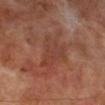illumination = cross-polarized illumination
imaging modality = 15 mm crop, total-body photography
body site = the leg
automated lesion analysis = border irregularity of about 5.5 on a 0–10 scale and a within-lesion color-variation index near 4/10; a nevus-likeness score of about 0/100 and lesion-presence confidence of about 100/100
lesion size = ≈4.5 mm
subject = male, aged around 70